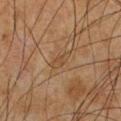biopsy status — no biopsy performed (imaged during a skin exam)
automated metrics — a border-irregularity rating of about 3/10, a within-lesion color-variation index near 1/10, and radial color variation of about 0.5; a lesion-detection confidence of about 95/100
image — ~15 mm crop, total-body skin-cancer survey
anatomic site — the chest
patient — male, aged around 50
illumination — cross-polarized illumination